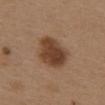  biopsy_status: not biopsied; imaged during a skin examination
  patient:
    sex: female
    age_approx: 40
  image:
    source: total-body photography crop
    field_of_view_mm: 15
  site: back